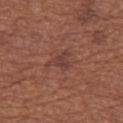Case summary:
* workup — catalogued during a skin exam; not biopsied
* site — the left thigh
* image — ~15 mm crop, total-body skin-cancer survey
* subject — female, about 55 years old